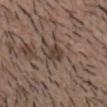Automated image analysis of the tile measured an area of roughly 6 mm², an eccentricity of roughly 0.65, and a shape-asymmetry score of about 0.25 (0 = symmetric). The software also gave a mean CIELAB color near L≈40 a*≈14 b*≈21 and a normalized lesion–skin contrast near 7. A close-up tile cropped from a whole-body skin photograph, about 15 mm across. The recorded lesion diameter is about 3.5 mm. Located on the head or neck. The tile uses white-light illumination. A male patient roughly 50 years of age.